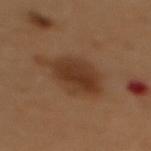Assessment:
This lesion was catalogued during total-body skin photography and was not selected for biopsy.
Background:
Longest diameter approximately 5 mm. On the back. The subject is a female approximately 50 years of age. Captured under cross-polarized illumination. Cropped from a total-body skin-imaging series; the visible field is about 15 mm.